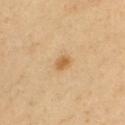biopsy status: no biopsy performed (imaged during a skin exam) | subject: male, aged 38–42 | tile lighting: cross-polarized | image-analysis metrics: a lesion area of about 3 mm² and a symmetry-axis asymmetry near 0.2; a lesion color around L≈60 a*≈20 b*≈41 in CIELAB and a lesion-to-skin contrast of about 7.5 (normalized; higher = more distinct); a classifier nevus-likeness of about 85/100 and lesion-presence confidence of about 100/100 | site: the upper back | size: about 2 mm | image: total-body-photography crop, ~15 mm field of view.